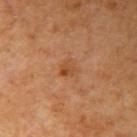Assessment:
The lesion was tiled from a total-body skin photograph and was not biopsied.
Acquisition and patient details:
A male patient, aged 58–62. On the right upper arm. A lesion tile, about 15 mm wide, cut from a 3D total-body photograph.